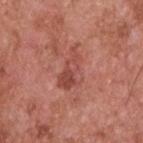Q: Was a biopsy performed?
A: total-body-photography surveillance lesion; no biopsy
Q: What are the patient's age and sex?
A: male, aged around 55
Q: Illumination type?
A: white-light illumination
Q: Lesion location?
A: the upper back
Q: Automated lesion metrics?
A: a footprint of about 8.5 mm², a shape eccentricity near 0.85, and a symmetry-axis asymmetry near 0.45; a mean CIELAB color near L≈49 a*≈28 b*≈28, about 8 CIELAB-L* units darker than the surrounding skin, and a normalized lesion–skin contrast near 6
Q: What is the imaging modality?
A: 15 mm crop, total-body photography